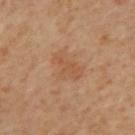Notes:
- notes · total-body-photography surveillance lesion; no biopsy